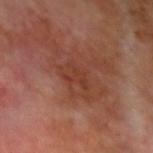The lesion was photographed on a routine skin check and not biopsied; there is no pathology result.
On the left upper arm.
Cropped from a whole-body photographic skin survey; the tile spans about 15 mm.
The lesion-visualizer software estimated an outline eccentricity of about 0.75 (0 = round, 1 = elongated) and two-axis asymmetry of about 0.45. The software also gave a border-irregularity rating of about 7/10, a within-lesion color-variation index near 0.5/10, and radial color variation of about 0. And it measured a detector confidence of about 100 out of 100 that the crop contains a lesion.
This is a cross-polarized tile.
A male subject, approximately 70 years of age.